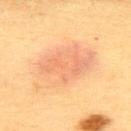biopsy_status: not biopsied; imaged during a skin examination
image:
  source: total-body photography crop
  field_of_view_mm: 15
site: right upper arm
automated_metrics:
  cielab_L: 64
  cielab_a: 22
  cielab_b: 36
  vs_skin_darker_L: 8.0
  vs_skin_contrast_norm: 5.5
  nevus_likeness_0_100: 30
  lesion_detection_confidence_0_100: 100
patient:
  sex: female
  age_approx: 55
lesion_size:
  long_diameter_mm_approx: 7.5